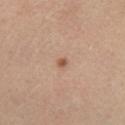The lesion was photographed on a routine skin check and not biopsied; there is no pathology result. A male patient aged approximately 40. This is a cross-polarized tile. About 1 mm across. A 15 mm crop from a total-body photograph taken for skin-cancer surveillance. From the leg.